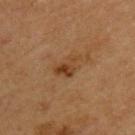follow-up: catalogued during a skin exam; not biopsied
acquisition: ~15 mm tile from a whole-body skin photo
lesion diameter: about 3.5 mm
location: the back
lighting: cross-polarized
patient: male, roughly 60 years of age
automated metrics: a footprint of about 5.5 mm², an outline eccentricity of about 0.8 (0 = round, 1 = elongated), and two-axis asymmetry of about 0.4; an average lesion color of about L≈36 a*≈18 b*≈32 (CIELAB), about 8 CIELAB-L* units darker than the surrounding skin, and a normalized lesion–skin contrast near 7.5; a border-irregularity index near 4.5/10, internal color variation of about 4.5 on a 0–10 scale, and a peripheral color-asymmetry measure near 1.5; lesion-presence confidence of about 100/100